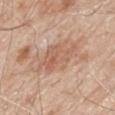<record>
<biopsy_status>not biopsied; imaged during a skin examination</biopsy_status>
<automated_metrics>
  <area_mm2_approx>13.0</area_mm2_approx>
  <shape_asymmetry>0.3</shape_asymmetry>
  <cielab_L>60</cielab_L>
  <cielab_a>20</cielab_a>
  <cielab_b>31</cielab_b>
  <vs_skin_darker_L>8.0</vs_skin_darker_L>
  <vs_skin_contrast_norm>5.5</vs_skin_contrast_norm>
  <border_irregularity_0_10>4.5</border_irregularity_0_10>
  <color_variation_0_10>3.5</color_variation_0_10>
  <peripheral_color_asymmetry>1.0</peripheral_color_asymmetry>
</automated_metrics>
<site>mid back</site>
<lesion_size>
  <long_diameter_mm_approx>6.0</long_diameter_mm_approx>
</lesion_size>
<image>
  <source>total-body photography crop</source>
  <field_of_view_mm>15</field_of_view_mm>
</image>
<patient>
  <sex>male</sex>
  <age_approx>80</age_approx>
</patient>
</record>